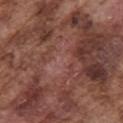biopsy status: catalogued during a skin exam; not biopsied | image: total-body-photography crop, ~15 mm field of view | anatomic site: the front of the torso | lesion diameter: about 17 mm | subject: male, approximately 75 years of age | TBP lesion metrics: a footprint of about 150 mm², a shape eccentricity near 0.55, and a shape-asymmetry score of about 0.4 (0 = symmetric); a lesion color around L≈40 a*≈21 b*≈23 in CIELAB and roughly 9 lightness units darker than nearby skin; a nevus-likeness score of about 0/100 and a lesion-detection confidence of about 90/100 | illumination: white-light illumination.